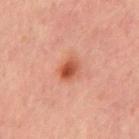The lesion was tiled from a total-body skin photograph and was not biopsied.
The lesion is on the mid back.
A 15 mm crop from a total-body photograph taken for skin-cancer surveillance.
The subject is a male aged 43 to 47.
The recorded lesion diameter is about 2.5 mm.
Imaged with cross-polarized lighting.